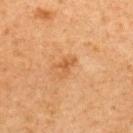biopsy_status: not biopsied; imaged during a skin examination
site: upper back
lesion_size:
  long_diameter_mm_approx: 2.5
patient:
  sex: female
  age_approx: 60
lighting: cross-polarized
image:
  source: total-body photography crop
  field_of_view_mm: 15